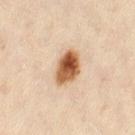| field | value |
|---|---|
| notes | imaged on a skin check; not biopsied |
| lighting | cross-polarized illumination |
| size | ~4 mm (longest diameter) |
| anatomic site | the lower back |
| image | ~15 mm tile from a whole-body skin photo |
| patient | female, aged 33 to 37 |